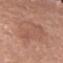Clinical impression:
This lesion was catalogued during total-body skin photography and was not selected for biopsy.
Clinical summary:
The recorded lesion diameter is about 10.5 mm. A female subject, in their mid-70s. From the head or neck. The tile uses white-light illumination. Automated tile analysis of the lesion measured border irregularity of about 5 on a 0–10 scale, a color-variation rating of about 3/10, and peripheral color asymmetry of about 1. The software also gave a classifier nevus-likeness of about 0/100 and a detector confidence of about 100 out of 100 that the crop contains a lesion. This image is a 15 mm lesion crop taken from a total-body photograph.